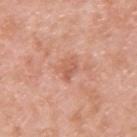{"patient": {"sex": "male", "age_approx": 40}, "automated_metrics": {"eccentricity": 0.75, "shape_asymmetry": 0.3, "cielab_L": 60, "cielab_a": 25, "cielab_b": 32, "border_irregularity_0_10": 3.0, "color_variation_0_10": 2.0, "peripheral_color_asymmetry": 1.0}, "image": {"source": "total-body photography crop", "field_of_view_mm": 15}, "lighting": "white-light", "lesion_size": {"long_diameter_mm_approx": 3.0}, "site": "upper back"}